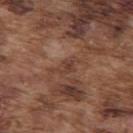Captured during whole-body skin photography for melanoma surveillance; the lesion was not biopsied. A male subject, aged approximately 75. The recorded lesion diameter is about 3 mm. From the upper back. Captured under white-light illumination. A roughly 15 mm field-of-view crop from a total-body skin photograph.